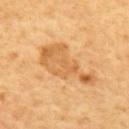Recorded during total-body skin imaging; not selected for excision or biopsy. A male patient roughly 60 years of age. On the back. This image is a 15 mm lesion crop taken from a total-body photograph. Approximately 7.5 mm at its widest. The lesion-visualizer software estimated a lesion color around L≈62 a*≈22 b*≈44 in CIELAB and a normalized border contrast of about 6.5. The software also gave a border-irregularity rating of about 6/10, a within-lesion color-variation index near 3.5/10, and a peripheral color-asymmetry measure near 0.5. The analysis additionally found lesion-presence confidence of about 100/100. This is a cross-polarized tile.